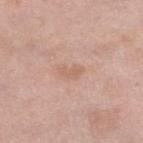Notes:
– biopsy status — imaged on a skin check; not biopsied
– subject — female, roughly 40 years of age
– illumination — white-light illumination
– automated lesion analysis — a lesion color around L≈62 a*≈20 b*≈29 in CIELAB, a lesion–skin lightness drop of about 7, and a normalized lesion–skin contrast near 5; a border-irregularity rating of about 4/10, a within-lesion color-variation index near 0/10, and peripheral color asymmetry of about 0; an automated nevus-likeness rating near 0 out of 100
– anatomic site — the right lower leg
– acquisition — 15 mm crop, total-body photography
– size — ≈2.5 mm